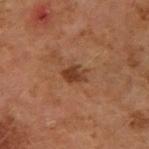Q: Was this lesion biopsied?
A: total-body-photography surveillance lesion; no biopsy
Q: What is the imaging modality?
A: total-body-photography crop, ~15 mm field of view
Q: What is the lesion's diameter?
A: ~2.5 mm (longest diameter)
Q: Patient demographics?
A: female, about 60 years old
Q: What is the anatomic site?
A: the left forearm
Q: Illumination type?
A: cross-polarized